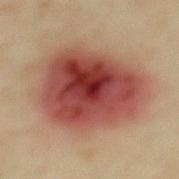workup: total-body-photography surveillance lesion; no biopsy
anatomic site: the back
lesion size: ≈9.5 mm
image: total-body-photography crop, ~15 mm field of view
subject: female, in their mid-30s
tile lighting: cross-polarized illumination